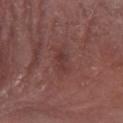Assessment:
Recorded during total-body skin imaging; not selected for excision or biopsy.
Image and clinical context:
The lesion-visualizer software estimated an area of roughly 3 mm², an outline eccentricity of about 0.9 (0 = round, 1 = elongated), and two-axis asymmetry of about 0.4. It also reported a lesion-to-skin contrast of about 5.5 (normalized; higher = more distinct). It also reported an automated nevus-likeness rating near 0 out of 100 and lesion-presence confidence of about 100/100. Longest diameter approximately 2.5 mm. The tile uses white-light illumination. Cropped from a total-body skin-imaging series; the visible field is about 15 mm. A male subject, aged approximately 80. The lesion is on the left forearm.